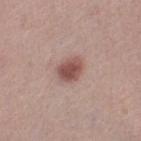A female subject aged approximately 40. The lesion is located on the left thigh. Approximately 3 mm at its widest. This image is a 15 mm lesion crop taken from a total-body photograph.